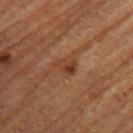Assessment:
The lesion was tiled from a total-body skin photograph and was not biopsied.
Background:
A 15 mm close-up tile from a total-body photography series done for melanoma screening. The tile uses cross-polarized illumination. About 3 mm across. The lesion is located on the left upper arm. A female patient aged 68–72.